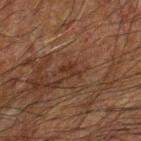This lesion was catalogued during total-body skin photography and was not selected for biopsy. Located on the left forearm. Captured under cross-polarized illumination. A 15 mm close-up extracted from a 3D total-body photography capture. Approximately 2.5 mm at its widest. The patient is a male aged 63–67.